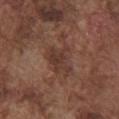biopsy status: imaged on a skin check; not biopsied | TBP lesion metrics: a footprint of about 8 mm², an outline eccentricity of about 0.65 (0 = round, 1 = elongated), and two-axis asymmetry of about 0.35; a mean CIELAB color near L≈36 a*≈19 b*≈24, roughly 7 lightness units darker than nearby skin, and a lesion-to-skin contrast of about 6.5 (normalized; higher = more distinct) | image: total-body-photography crop, ~15 mm field of view | lighting: white-light illumination | site: the chest | lesion size: about 3.5 mm | subject: male, aged approximately 75.